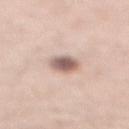Captured during whole-body skin photography for melanoma surveillance; the lesion was not biopsied. The total-body-photography lesion software estimated about 16 CIELAB-L* units darker than the surrounding skin. It also reported a classifier nevus-likeness of about 65/100 and a detector confidence of about 100 out of 100 that the crop contains a lesion. Captured under white-light illumination. A male subject, in their 60s. On the front of the torso. The recorded lesion diameter is about 3 mm. A 15 mm crop from a total-body photograph taken for skin-cancer surveillance.